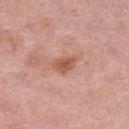| feature | finding |
|---|---|
| workup | total-body-photography surveillance lesion; no biopsy |
| automated metrics | a mean CIELAB color near L≈56 a*≈26 b*≈29, roughly 10 lightness units darker than nearby skin, and a normalized border contrast of about 7.5; a classifier nevus-likeness of about 15/100 and a detector confidence of about 100 out of 100 that the crop contains a lesion |
| image | 15 mm crop, total-body photography |
| lighting | white-light illumination |
| site | the leg |
| subject | female, aged around 40 |